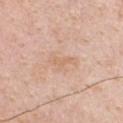Q: Is there a histopathology result?
A: catalogued during a skin exam; not biopsied
Q: How was the tile lit?
A: white-light
Q: Lesion location?
A: the front of the torso
Q: Automated lesion metrics?
A: an automated nevus-likeness rating near 0 out of 100 and a lesion-detection confidence of about 100/100
Q: Lesion size?
A: ≈3.5 mm
Q: Patient demographics?
A: male, aged approximately 50
Q: What is the imaging modality?
A: 15 mm crop, total-body photography